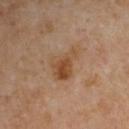| feature | finding |
|---|---|
| workup | catalogued during a skin exam; not biopsied |
| lesion diameter | ≈4.5 mm |
| body site | the left upper arm |
| tile lighting | cross-polarized illumination |
| image | ~15 mm crop, total-body skin-cancer survey |
| subject | male, roughly 55 years of age |